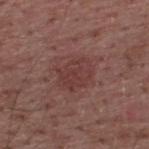biopsy status: imaged on a skin check; not biopsied | lighting: white-light | size: ≈4 mm | image: ~15 mm tile from a whole-body skin photo | location: the upper back | subject: male, roughly 55 years of age.